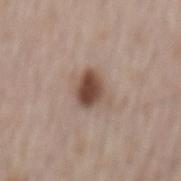This lesion was catalogued during total-body skin photography and was not selected for biopsy. A male patient approximately 65 years of age. The lesion is on the back. Cropped from a whole-body photographic skin survey; the tile spans about 15 mm. The recorded lesion diameter is about 3.5 mm.